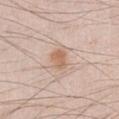Longest diameter approximately 2.5 mm. A 15 mm crop from a total-body photograph taken for skin-cancer surveillance. The lesion is located on the abdomen. A male patient aged approximately 35.